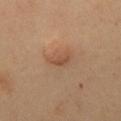follow-up=total-body-photography surveillance lesion; no biopsy
acquisition=total-body-photography crop, ~15 mm field of view
lighting=cross-polarized illumination
patient=male, in their 50s
diameter=~2.5 mm (longest diameter)
body site=the chest